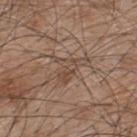Case summary:
• follow-up: no biopsy performed (imaged during a skin exam)
• acquisition: total-body-photography crop, ~15 mm field of view
• illumination: white-light
• lesion diameter: ≈4.5 mm
• automated metrics: a mean CIELAB color near L≈47 a*≈16 b*≈26 and about 7 CIELAB-L* units darker than the surrounding skin; border irregularity of about 8.5 on a 0–10 scale and radial color variation of about 1; a classifier nevus-likeness of about 5/100 and a detector confidence of about 70 out of 100 that the crop contains a lesion
• subject: male, aged approximately 45
• site: the upper back